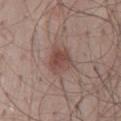Assessment: This lesion was catalogued during total-body skin photography and was not selected for biopsy. Background: A 15 mm close-up extracted from a 3D total-body photography capture. About 4 mm across. The tile uses white-light illumination. A male subject aged approximately 50. On the mid back.